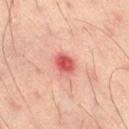| key | value |
|---|---|
| workup | imaged on a skin check; not biopsied |
| size | ~2.5 mm (longest diameter) |
| image source | total-body-photography crop, ~15 mm field of view |
| patient | male, in their mid- to late 30s |
| lighting | cross-polarized |
| anatomic site | the right thigh |